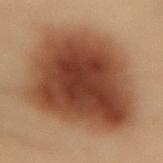Q: Is there a histopathology result?
A: imaged on a skin check; not biopsied
Q: What kind of image is this?
A: ~15 mm crop, total-body skin-cancer survey
Q: Lesion size?
A: about 11 mm
Q: Automated lesion metrics?
A: a mean CIELAB color near L≈35 a*≈19 b*≈26, roughly 14 lightness units darker than nearby skin, and a lesion-to-skin contrast of about 12 (normalized; higher = more distinct); a border-irregularity index near 2/10, a color-variation rating of about 6/10, and peripheral color asymmetry of about 1.5; an automated nevus-likeness rating near 100 out of 100 and lesion-presence confidence of about 100/100
Q: Who is the patient?
A: male, in their mid- to late 50s
Q: What is the anatomic site?
A: the lower back
Q: Illumination type?
A: cross-polarized illumination